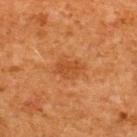The lesion was photographed on a routine skin check and not biopsied; there is no pathology result. Approximately 3.5 mm at its widest. A close-up tile cropped from a whole-body skin photograph, about 15 mm across. Located on the upper back. A male patient aged around 65. Automated tile analysis of the lesion measured roughly 6 lightness units darker than nearby skin and a normalized lesion–skin contrast near 5.5. It also reported a border-irregularity index near 3/10 and a peripheral color-asymmetry measure near 0.5. The software also gave a classifier nevus-likeness of about 30/100 and a lesion-detection confidence of about 100/100. Imaged with cross-polarized lighting.